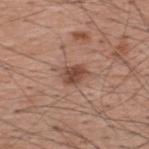Findings:
– follow-up: catalogued during a skin exam; not biopsied
– size: ~3 mm (longest diameter)
– patient: male, about 60 years old
– location: the upper back
– tile lighting: white-light illumination
– image source: ~15 mm crop, total-body skin-cancer survey
– TBP lesion metrics: a footprint of about 5 mm² and a shape eccentricity near 0.7